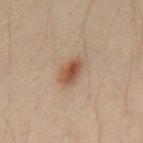| field | value |
|---|---|
| anatomic site | the chest |
| illumination | cross-polarized |
| image-analysis metrics | an area of roughly 6 mm², an outline eccentricity of about 0.7 (0 = round, 1 = elongated), and two-axis asymmetry of about 0.15; a nevus-likeness score of about 95/100 and a lesion-detection confidence of about 100/100 |
| image source | 15 mm crop, total-body photography |
| subject | male, about 30 years old |